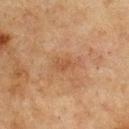{
  "patient": {
    "sex": "male",
    "age_approx": 75
  },
  "automated_metrics": {
    "vs_skin_contrast_norm": 5.0,
    "border_irregularity_0_10": 3.5,
    "peripheral_color_asymmetry": 0.5,
    "lesion_detection_confidence_0_100": 100
  },
  "site": "chest",
  "lesion_size": {
    "long_diameter_mm_approx": 3.5
  },
  "image": {
    "source": "total-body photography crop",
    "field_of_view_mm": 15
  }
}